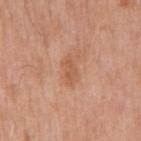On the mid back. The lesion's longest dimension is about 3.5 mm. A male patient, aged approximately 75. Automated image analysis of the tile measured a lesion area of about 4.5 mm² and an eccentricity of roughly 0.85. And it measured a lesion color around L≈57 a*≈24 b*≈34 in CIELAB and about 8 CIELAB-L* units darker than the surrounding skin. The analysis additionally found a border-irregularity index near 3/10, a within-lesion color-variation index near 1.5/10, and a peripheral color-asymmetry measure near 0.5. The analysis additionally found an automated nevus-likeness rating near 0 out of 100. A lesion tile, about 15 mm wide, cut from a 3D total-body photograph.